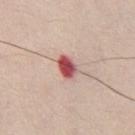workup = no biopsy performed (imaged during a skin exam); diameter = ~2.5 mm (longest diameter); subject = male, aged 33 to 37; lighting = white-light illumination; image source = ~15 mm crop, total-body skin-cancer survey; anatomic site = the front of the torso.